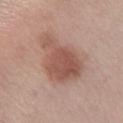  biopsy_status: not biopsied; imaged during a skin examination
  lesion_size:
    long_diameter_mm_approx: 6.5
  patient:
    sex: female
    age_approx: 55
  site: leg
  image:
    source: total-body photography crop
    field_of_view_mm: 15
  automated_metrics:
    area_mm2_approx: 21.0
    eccentricity: 0.7
    shape_asymmetry: 0.4
    border_irregularity_0_10: 4.5
    color_variation_0_10: 4.0
    peripheral_color_asymmetry: 1.0
    nevus_likeness_0_100: 90
    lesion_detection_confidence_0_100: 100